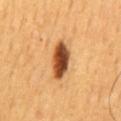biopsy status — total-body-photography surveillance lesion; no biopsy
diameter — about 5 mm
acquisition — total-body-photography crop, ~15 mm field of view
subject — male, about 60 years old
body site — the mid back
illumination — cross-polarized illumination
automated metrics — a footprint of about 9.5 mm², an outline eccentricity of about 0.85 (0 = round, 1 = elongated), and a shape-asymmetry score of about 0.15 (0 = symmetric); border irregularity of about 2 on a 0–10 scale, internal color variation of about 8.5 on a 0–10 scale, and peripheral color asymmetry of about 2.5